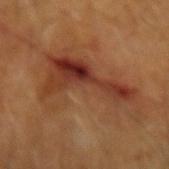Q: How large is the lesion?
A: about 9.5 mm
Q: How was the tile lit?
A: cross-polarized
Q: Patient demographics?
A: male, aged approximately 65
Q: What kind of image is this?
A: 15 mm crop, total-body photography